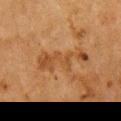Part of a total-body skin-imaging series; this lesion was reviewed on a skin check and was not flagged for biopsy. A female patient aged approximately 50. The tile uses cross-polarized illumination. On the chest. This image is a 15 mm lesion crop taken from a total-body photograph.